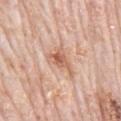Case summary:
– notes · catalogued during a skin exam; not biopsied
– acquisition · ~15 mm crop, total-body skin-cancer survey
– diameter · about 4 mm
– TBP lesion metrics · a mean CIELAB color near L≈62 a*≈22 b*≈32, roughly 10 lightness units darker than nearby skin, and a lesion-to-skin contrast of about 7 (normalized; higher = more distinct); a border-irregularity index near 6/10, a color-variation rating of about 6/10, and peripheral color asymmetry of about 2
– body site · the mid back
– lighting · white-light
– patient · male, about 80 years old